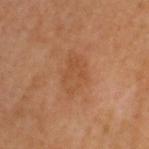Part of a total-body skin-imaging series; this lesion was reviewed on a skin check and was not flagged for biopsy. A male patient, in their mid-50s. A lesion tile, about 15 mm wide, cut from a 3D total-body photograph. On the head or neck.